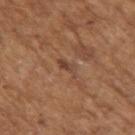Q: Was this lesion biopsied?
A: total-body-photography surveillance lesion; no biopsy